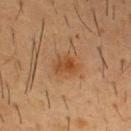{
  "biopsy_status": "not biopsied; imaged during a skin examination",
  "lighting": "cross-polarized",
  "site": "chest",
  "lesion_size": {
    "long_diameter_mm_approx": 3.0
  },
  "image": {
    "source": "total-body photography crop",
    "field_of_view_mm": 15
  },
  "patient": {
    "sex": "male",
    "age_approx": 55
  }
}